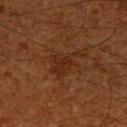Recorded during total-body skin imaging; not selected for excision or biopsy. Longest diameter approximately 4 mm. The lesion is located on the right upper arm. A roughly 15 mm field-of-view crop from a total-body skin photograph. This is a cross-polarized tile. A male subject aged 58–62.